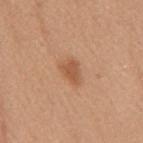• biopsy status: imaged on a skin check; not biopsied
• acquisition: total-body-photography crop, ~15 mm field of view
• location: the mid back
• patient: female, aged approximately 40
• lesion size: about 3 mm
• TBP lesion metrics: an area of roughly 4.5 mm², an outline eccentricity of about 0.75 (0 = round, 1 = elongated), and a shape-asymmetry score of about 0.3 (0 = symmetric); a mean CIELAB color near L≈55 a*≈23 b*≈35, a lesion–skin lightness drop of about 9, and a normalized lesion–skin contrast near 6.5
• lighting: white-light illumination